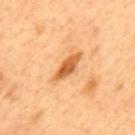Case summary:
* workup — total-body-photography surveillance lesion; no biopsy
* size — ~4.5 mm (longest diameter)
* tile lighting — cross-polarized
* subject — male, in their 50s
* image source — 15 mm crop, total-body photography
* body site — the mid back